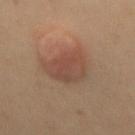{"biopsy_status": "not biopsied; imaged during a skin examination", "image": {"source": "total-body photography crop", "field_of_view_mm": 15}, "patient": {"sex": "female", "age_approx": 50}, "lesion_size": {"long_diameter_mm_approx": 4.5}, "site": "back"}